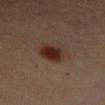| field | value |
|---|---|
| biopsy status | imaged on a skin check; not biopsied |
| patient | female, aged 33 to 37 |
| anatomic site | the left forearm |
| automated lesion analysis | a footprint of about 9 mm², a shape eccentricity near 0.35, and two-axis asymmetry of about 0.2; roughly 10 lightness units darker than nearby skin and a normalized border contrast of about 10.5; border irregularity of about 2 on a 0–10 scale and internal color variation of about 4 on a 0–10 scale |
| acquisition | total-body-photography crop, ~15 mm field of view |
| illumination | cross-polarized illumination |
| lesion size | ≈3.5 mm |